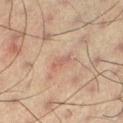notes: no biopsy performed (imaged during a skin exam) | automated lesion analysis: a lesion area of about 2 mm², an outline eccentricity of about 0.95 (0 = round, 1 = elongated), and a symmetry-axis asymmetry near 0.4; a mean CIELAB color near L≈51 a*≈19 b*≈25, roughly 7 lightness units darker than nearby skin, and a lesion-to-skin contrast of about 5 (normalized; higher = more distinct) | subject: male, aged 58–62 | image source: ~15 mm tile from a whole-body skin photo | lesion diameter: ≈2.5 mm | tile lighting: cross-polarized illumination.